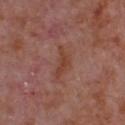Q: Was this lesion biopsied?
A: no biopsy performed (imaged during a skin exam)
Q: Lesion size?
A: about 4 mm
Q: Illumination type?
A: white-light illumination
Q: How was this image acquired?
A: total-body-photography crop, ~15 mm field of view
Q: Where on the body is the lesion?
A: the chest
Q: Patient demographics?
A: male, about 65 years old
Q: Automated lesion metrics?
A: an average lesion color of about L≈42 a*≈24 b*≈29 (CIELAB), roughly 7 lightness units darker than nearby skin, and a normalized border contrast of about 7.5; a border-irregularity index near 7/10, a color-variation rating of about 0.5/10, and radial color variation of about 0; a nevus-likeness score of about 0/100 and lesion-presence confidence of about 95/100